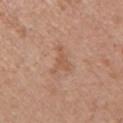Background:
A roughly 15 mm field-of-view crop from a total-body skin photograph. The total-body-photography lesion software estimated a mean CIELAB color near L≈56 a*≈21 b*≈31, a lesion–skin lightness drop of about 7, and a lesion-to-skin contrast of about 5.5 (normalized; higher = more distinct). The software also gave a border-irregularity rating of about 7.5/10, a within-lesion color-variation index near 1.5/10, and a peripheral color-asymmetry measure near 0.5. And it measured an automated nevus-likeness rating near 0 out of 100 and a lesion-detection confidence of about 100/100. From the right upper arm. This is a white-light tile. The patient is a female aged 58–62.